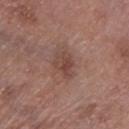The lesion is on the left lower leg.
A male subject in their 80s.
This is a white-light tile.
Cropped from a whole-body photographic skin survey; the tile spans about 15 mm.
The recorded lesion diameter is about 3.5 mm.